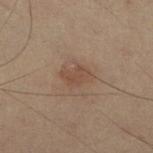Q: Is there a histopathology result?
A: catalogued during a skin exam; not biopsied
Q: How was the tile lit?
A: cross-polarized
Q: Who is the patient?
A: male, in their mid- to late 50s
Q: What is the imaging modality?
A: ~15 mm tile from a whole-body skin photo
Q: What is the lesion's diameter?
A: ~3.5 mm (longest diameter)
Q: Lesion location?
A: the right lower leg